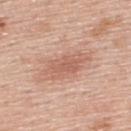Impression:
Recorded during total-body skin imaging; not selected for excision or biopsy.
Context:
The patient is a male in their mid- to late 50s. A close-up tile cropped from a whole-body skin photograph, about 15 mm across. The lesion is on the back.